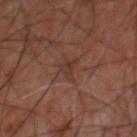Q: Was this lesion biopsied?
A: catalogued during a skin exam; not biopsied
Q: Lesion size?
A: ~3 mm (longest diameter)
Q: Lesion location?
A: the upper back
Q: Automated lesion metrics?
A: a symmetry-axis asymmetry near 0.35; an average lesion color of about L≈31 a*≈17 b*≈22 (CIELAB), about 5 CIELAB-L* units darker than the surrounding skin, and a normalized lesion–skin contrast near 5; an automated nevus-likeness rating near 0 out of 100
Q: Patient demographics?
A: male, approximately 60 years of age
Q: What is the imaging modality?
A: total-body-photography crop, ~15 mm field of view